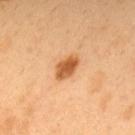Assessment: The lesion was photographed on a routine skin check and not biopsied; there is no pathology result. Context: The subject is a male aged 48 to 52. On the back. Cropped from a whole-body photographic skin survey; the tile spans about 15 mm.